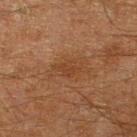biopsy status: total-body-photography surveillance lesion; no biopsy | patient: male, aged approximately 60 | acquisition: total-body-photography crop, ~15 mm field of view | image-analysis metrics: a footprint of about 4 mm² and two-axis asymmetry of about 0.4; a color-variation rating of about 2/10 and peripheral color asymmetry of about 1 | tile lighting: cross-polarized | body site: the right lower leg | size: about 3 mm.